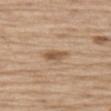location: the left thigh | patient: male, aged around 70 | illumination: white-light illumination | imaging modality: ~15 mm tile from a whole-body skin photo.